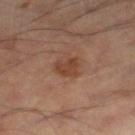workup=catalogued during a skin exam; not biopsied
automated lesion analysis=an eccentricity of roughly 0.6 and a symmetry-axis asymmetry near 0.35; a normalized border contrast of about 7.5
acquisition=~15 mm crop, total-body skin-cancer survey
body site=the right lower leg
size=about 2.5 mm
lighting=cross-polarized illumination
patient=male, in their mid-50s